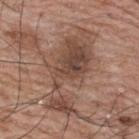Impression: Recorded during total-body skin imaging; not selected for excision or biopsy.